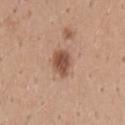Background: A male subject aged 38 to 42. On the back. A 15 mm close-up extracted from a 3D total-body photography capture.